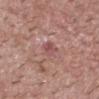Clinical impression:
The lesion was photographed on a routine skin check and not biopsied; there is no pathology result.
Context:
A male subject, aged around 25. A close-up tile cropped from a whole-body skin photograph, about 15 mm across. The tile uses white-light illumination. The lesion is located on the head or neck. The recorded lesion diameter is about 3 mm.